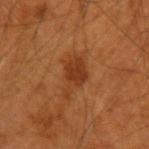workup = total-body-photography surveillance lesion; no biopsy | size = about 5 mm | automated lesion analysis = a border-irregularity rating of about 5.5/10 and a color-variation rating of about 2/10 | site = the left forearm | patient = male, approximately 55 years of age | acquisition = ~15 mm tile from a whole-body skin photo | illumination = cross-polarized illumination.